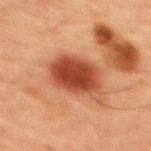A close-up tile cropped from a whole-body skin photograph, about 15 mm across.
The patient is a male roughly 60 years of age.
Located on the upper back.